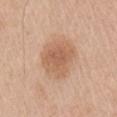Q: Is there a histopathology result?
A: no biopsy performed (imaged during a skin exam)
Q: Who is the patient?
A: male, aged 63 to 67
Q: Lesion size?
A: ≈5 mm
Q: What is the anatomic site?
A: the arm
Q: What is the imaging modality?
A: total-body-photography crop, ~15 mm field of view